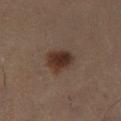Case summary:
• notes: catalogued during a skin exam; not biopsied
• subject: female, in their 50s
• image source: total-body-photography crop, ~15 mm field of view
• location: the left thigh